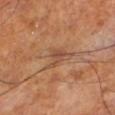<case>
  <biopsy_status>not biopsied; imaged during a skin examination</biopsy_status>
  <lesion_size>
    <long_diameter_mm_approx>2.5</long_diameter_mm_approx>
  </lesion_size>
  <site>left lower leg</site>
  <patient>
    <sex>male</sex>
    <age_approx>70</age_approx>
  </patient>
  <lighting>cross-polarized</lighting>
  <image>
    <source>total-body photography crop</source>
    <field_of_view_mm>15</field_of_view_mm>
  </image>
</case>